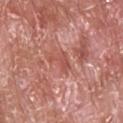Findings:
- follow-up — no biopsy performed (imaged during a skin exam)
- patient — male, aged 63 to 67
- image — ~15 mm crop, total-body skin-cancer survey
- location — the head or neck
- illumination — white-light
- size — ≈3 mm